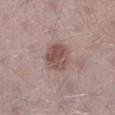<record>
<biopsy_status>not biopsied; imaged during a skin examination</biopsy_status>
<lesion_size>
  <long_diameter_mm_approx>3.5</long_diameter_mm_approx>
</lesion_size>
<patient>
  <sex>male</sex>
  <age_approx>40</age_approx>
</patient>
<automated_metrics>
  <eccentricity>0.55</eccentricity>
  <shape_asymmetry>0.15</shape_asymmetry>
</automated_metrics>
<lighting>white-light</lighting>
<image>
  <source>total-body photography crop</source>
  <field_of_view_mm>15</field_of_view_mm>
</image>
<site>leg</site>
</record>